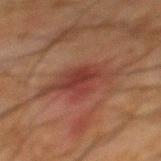Q: Was a biopsy performed?
A: imaged on a skin check; not biopsied
Q: Lesion size?
A: ~7 mm (longest diameter)
Q: Where on the body is the lesion?
A: the mid back
Q: What are the patient's age and sex?
A: male, in their mid- to late 60s
Q: How was this image acquired?
A: 15 mm crop, total-body photography
Q: Illumination type?
A: cross-polarized illumination